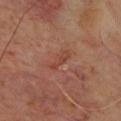Q: Is there a histopathology result?
A: imaged on a skin check; not biopsied
Q: What is the anatomic site?
A: the chest
Q: What are the patient's age and sex?
A: male, in their mid- to late 60s
Q: What lighting was used for the tile?
A: cross-polarized
Q: Lesion size?
A: ~3 mm (longest diameter)
Q: What kind of image is this?
A: ~15 mm tile from a whole-body skin photo